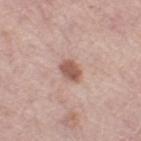{
  "biopsy_status": "not biopsied; imaged during a skin examination",
  "lighting": "white-light",
  "lesion_size": {
    "long_diameter_mm_approx": 3.0
  },
  "site": "left thigh",
  "automated_metrics": {
    "area_mm2_approx": 4.0,
    "shape_asymmetry": 0.25
  },
  "patient": {
    "sex": "female",
    "age_approx": 65
  },
  "image": {
    "source": "total-body photography crop",
    "field_of_view_mm": 15
  }
}